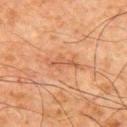* notes — total-body-photography surveillance lesion; no biopsy
* imaging modality — 15 mm crop, total-body photography
* lesion size — ≈4 mm
* automated metrics — a lesion area of about 3.5 mm², an outline eccentricity of about 0.95 (0 = round, 1 = elongated), and two-axis asymmetry of about 0.45
* patient — male, roughly 65 years of age
* lighting — cross-polarized
* location — the right upper arm